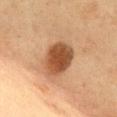Imaged during a routine full-body skin examination; the lesion was not biopsied and no histopathology is available. The patient is a female approximately 50 years of age. Longest diameter approximately 4.5 mm. From the chest. A lesion tile, about 15 mm wide, cut from a 3D total-body photograph.